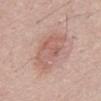The lesion was photographed on a routine skin check and not biopsied; there is no pathology result.
Located on the abdomen.
A roughly 15 mm field-of-view crop from a total-body skin photograph.
A male patient, in their 50s.
Approximately 6 mm at its widest.